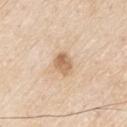On the arm. This image is a 15 mm lesion crop taken from a total-body photograph. A male subject, in their 80s. An algorithmic analysis of the crop reported a footprint of about 5.5 mm², a shape eccentricity near 0.65, and a symmetry-axis asymmetry near 0.25. The analysis additionally found a border-irregularity index near 2/10 and peripheral color asymmetry of about 1.5. The analysis additionally found a classifier nevus-likeness of about 30/100 and lesion-presence confidence of about 100/100.